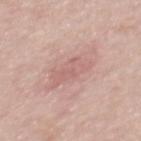Notes:
• follow-up · imaged on a skin check; not biopsied
• body site · the mid back
• patient · male, aged approximately 65
• diameter · about 6 mm
• image · ~15 mm crop, total-body skin-cancer survey